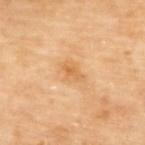The subject is a female aged approximately 65. Measured at roughly 3 mm in maximum diameter. A roughly 15 mm field-of-view crop from a total-body skin photograph. Automated tile analysis of the lesion measured two-axis asymmetry of about 0.3. The software also gave internal color variation of about 3 on a 0–10 scale. The analysis additionally found an automated nevus-likeness rating near 0 out of 100 and a detector confidence of about 100 out of 100 that the crop contains a lesion. Located on the upper back.